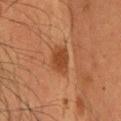{
  "biopsy_status": "not biopsied; imaged during a skin examination",
  "site": "head or neck",
  "lesion_size": {
    "long_diameter_mm_approx": 3.0
  },
  "automated_metrics": {
    "area_mm2_approx": 6.5,
    "eccentricity": 0.65,
    "shape_asymmetry": 0.15,
    "vs_skin_darker_L": 9.0,
    "vs_skin_contrast_norm": 7.5
  },
  "patient": {
    "sex": "male",
    "age_approx": 35
  },
  "image": {
    "source": "total-body photography crop",
    "field_of_view_mm": 15
  }
}